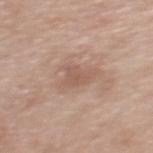Recorded during total-body skin imaging; not selected for excision or biopsy.
Imaged with white-light lighting.
This image is a 15 mm lesion crop taken from a total-body photograph.
The patient is a female aged 38 to 42.
The lesion's longest dimension is about 3 mm.
The lesion is located on the upper back.